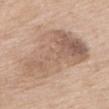Image and clinical context: The lesion is on the mid back. The tile uses white-light illumination. This image is a 15 mm lesion crop taken from a total-body photograph. A female patient aged approximately 75. Automated tile analysis of the lesion measured a shape eccentricity near 0.9 and a shape-asymmetry score of about 0.35 (0 = symmetric).